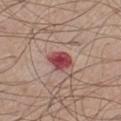Impression: The lesion was photographed on a routine skin check and not biopsied; there is no pathology result. Acquisition and patient details: A male subject, roughly 65 years of age. An algorithmic analysis of the crop reported an area of roughly 6 mm², an eccentricity of roughly 0.65, and a shape-asymmetry score of about 0.2 (0 = symmetric). The software also gave a mean CIELAB color near L≈46 a*≈32 b*≈22, a lesion–skin lightness drop of about 15, and a normalized lesion–skin contrast near 11. The software also gave a nevus-likeness score of about 0/100 and a detector confidence of about 100 out of 100 that the crop contains a lesion. A 15 mm close-up tile from a total-body photography series done for melanoma screening. The tile uses white-light illumination. The lesion's longest dimension is about 3 mm. From the left thigh.